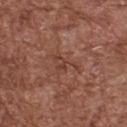<record>
<biopsy_status>not biopsied; imaged during a skin examination</biopsy_status>
<site>back</site>
<patient>
  <sex>male</sex>
  <age_approx>75</age_approx>
</patient>
<lighting>white-light</lighting>
<lesion_size>
  <long_diameter_mm_approx>4.0</long_diameter_mm_approx>
</lesion_size>
<image>
  <source>total-body photography crop</source>
  <field_of_view_mm>15</field_of_view_mm>
</image>
<automated_metrics>
  <area_mm2_approx>4.0</area_mm2_approx>
  <shape_asymmetry>0.6</shape_asymmetry>
  <cielab_L>41</cielab_L>
  <cielab_a>23</cielab_a>
  <cielab_b>27</cielab_b>
  <vs_skin_darker_L>7.0</vs_skin_darker_L>
  <border_irregularity_0_10>7.5</border_irregularity_0_10>
</automated_metrics>
</record>